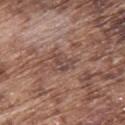Clinical summary: An algorithmic analysis of the crop reported a mean CIELAB color near L≈45 a*≈19 b*≈23, about 7 CIELAB-L* units darker than the surrounding skin, and a normalized border contrast of about 6. It also reported a nevus-likeness score of about 0/100 and lesion-presence confidence of about 95/100. This image is a 15 mm lesion crop taken from a total-body photograph. The lesion's longest dimension is about 2.5 mm. The tile uses white-light illumination. The lesion is on the upper back. A male patient in their mid- to late 70s.